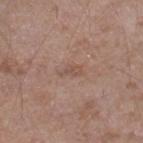The lesion was tiled from a total-body skin photograph and was not biopsied.